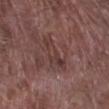| field | value |
|---|---|
| notes | imaged on a skin check; not biopsied |
| imaging modality | ~15 mm tile from a whole-body skin photo |
| anatomic site | the right lower leg |
| subject | male, approximately 80 years of age |
| lesion diameter | about 4.5 mm |
| lighting | white-light illumination |